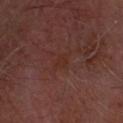The lesion was photographed on a routine skin check and not biopsied; there is no pathology result. A male patient, aged 58 to 62. The recorded lesion diameter is about 2.5 mm. The lesion-visualizer software estimated a lesion area of about 3.5 mm² and a symmetry-axis asymmetry near 0.4. Cropped from a whole-body photographic skin survey; the tile spans about 15 mm. From the head or neck.